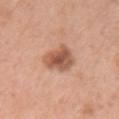Part of a total-body skin-imaging series; this lesion was reviewed on a skin check and was not flagged for biopsy. Automated tile analysis of the lesion measured a mean CIELAB color near L≈56 a*≈24 b*≈32, roughly 13 lightness units darker than nearby skin, and a lesion-to-skin contrast of about 8.5 (normalized; higher = more distinct). It also reported lesion-presence confidence of about 100/100. This is a white-light tile. Measured at roughly 4 mm in maximum diameter. This image is a 15 mm lesion crop taken from a total-body photograph. A female subject, in their mid-40s. The lesion is located on the chest.